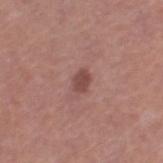Clinical impression:
Imaged during a routine full-body skin examination; the lesion was not biopsied and no histopathology is available.
Acquisition and patient details:
The lesion's longest dimension is about 2.5 mm. The lesion is located on the right thigh. Cropped from a whole-body photographic skin survey; the tile spans about 15 mm. The patient is a female roughly 50 years of age. Imaged with white-light lighting.